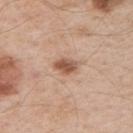Impression: No biopsy was performed on this lesion — it was imaged during a full skin examination and was not determined to be concerning. Background: A lesion tile, about 15 mm wide, cut from a 3D total-body photograph. Automated image analysis of the tile measured a mean CIELAB color near L≈55 a*≈21 b*≈31, about 13 CIELAB-L* units darker than the surrounding skin, and a normalized border contrast of about 8.5. It also reported border irregularity of about 1.5 on a 0–10 scale, a color-variation rating of about 3.5/10, and peripheral color asymmetry of about 1. And it measured an automated nevus-likeness rating near 90 out of 100 and lesion-presence confidence of about 100/100. Measured at roughly 2.5 mm in maximum diameter. Captured under white-light illumination. The lesion is located on the arm. A male patient aged 53–57.